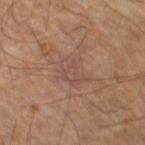Imaged during a routine full-body skin examination; the lesion was not biopsied and no histopathology is available. A 15 mm close-up tile from a total-body photography series done for melanoma screening. A male subject, aged 53–57. An algorithmic analysis of the crop reported a lesion area of about 6 mm² and two-axis asymmetry of about 0.35. The analysis additionally found radial color variation of about 1. It also reported a classifier nevus-likeness of about 0/100 and a lesion-detection confidence of about 90/100. The tile uses cross-polarized illumination. The lesion's longest dimension is about 4 mm. On the left lower leg.